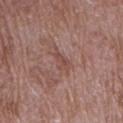Q: Was this lesion biopsied?
A: imaged on a skin check; not biopsied
Q: Illumination type?
A: white-light illumination
Q: How was this image acquired?
A: ~15 mm tile from a whole-body skin photo
Q: Automated lesion metrics?
A: a border-irregularity index near 4/10, a within-lesion color-variation index near 2/10, and a peripheral color-asymmetry measure near 1; a classifier nevus-likeness of about 0/100 and a lesion-detection confidence of about 70/100
Q: Where on the body is the lesion?
A: the right upper arm
Q: Who is the patient?
A: female, approximately 70 years of age
Q: How large is the lesion?
A: ~3 mm (longest diameter)